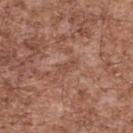Assessment: The lesion was tiled from a total-body skin photograph and was not biopsied. Clinical summary: Approximately 2.5 mm at its widest. The tile uses white-light illumination. The lesion is located on the upper back. A 15 mm close-up extracted from a 3D total-body photography capture. The subject is a male in their mid- to late 50s. The lesion-visualizer software estimated roughly 6 lightness units darker than nearby skin. The software also gave a border-irregularity rating of about 4.5/10, a color-variation rating of about 0/10, and radial color variation of about 0.